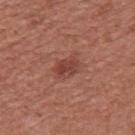The lesion was tiled from a total-body skin photograph and was not biopsied.
On the chest.
Captured under white-light illumination.
The lesion's longest dimension is about 3 mm.
A 15 mm crop from a total-body photograph taken for skin-cancer surveillance.
A male subject approximately 45 years of age.